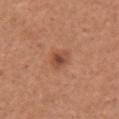This lesion was catalogued during total-body skin photography and was not selected for biopsy. The lesion's longest dimension is about 2.5 mm. An algorithmic analysis of the crop reported an average lesion color of about L≈49 a*≈25 b*≈32 (CIELAB) and a lesion-to-skin contrast of about 7 (normalized; higher = more distinct). Imaged with white-light lighting. A region of skin cropped from a whole-body photographic capture, roughly 15 mm wide. On the arm. A female subject, aged around 55.